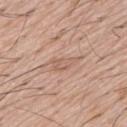No biopsy was performed on this lesion — it was imaged during a full skin examination and was not determined to be concerning. Approximately 4 mm at its widest. The lesion-visualizer software estimated a lesion area of about 5.5 mm², an outline eccentricity of about 0.9 (0 = round, 1 = elongated), and a shape-asymmetry score of about 0.4 (0 = symmetric). The software also gave a nevus-likeness score of about 0/100 and lesion-presence confidence of about 60/100. This image is a 15 mm lesion crop taken from a total-body photograph. Imaged with white-light lighting. A male patient in their mid-50s.